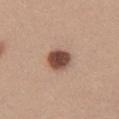– workup · no biopsy performed (imaged during a skin exam)
– subject · female, approximately 25 years of age
– site · the chest
– imaging modality · ~15 mm crop, total-body skin-cancer survey